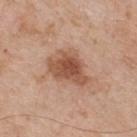Imaged during a routine full-body skin examination; the lesion was not biopsied and no histopathology is available.